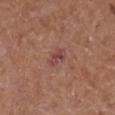Assessment:
No biopsy was performed on this lesion — it was imaged during a full skin examination and was not determined to be concerning.
Background:
A male subject, roughly 75 years of age. Captured under white-light illumination. Cropped from a total-body skin-imaging series; the visible field is about 15 mm. Located on the right lower leg. An algorithmic analysis of the crop reported a detector confidence of about 100 out of 100 that the crop contains a lesion. Measured at roughly 2.5 mm in maximum diameter.